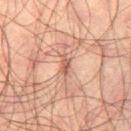This is a cross-polarized tile.
About 3 mm across.
A close-up tile cropped from a whole-body skin photograph, about 15 mm across.
From the right thigh.
A male subject, aged around 50.